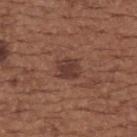• subject — female, aged 63 to 67
• automated lesion analysis — a lesion area of about 6.5 mm², an outline eccentricity of about 0.55 (0 = round, 1 = elongated), and two-axis asymmetry of about 0.2; a border-irregularity index near 2/10 and radial color variation of about 0.5; a nevus-likeness score of about 50/100
• lighting — white-light illumination
• image — 15 mm crop, total-body photography
• location — the upper back